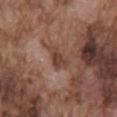{
  "image": {
    "source": "total-body photography crop",
    "field_of_view_mm": 15
  },
  "patient": {
    "sex": "male",
    "age_approx": 75
  },
  "site": "back"
}